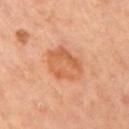No biopsy was performed on this lesion — it was imaged during a full skin examination and was not determined to be concerning. Located on the right upper arm. A roughly 15 mm field-of-view crop from a total-body skin photograph. A female subject, in their mid- to late 30s.